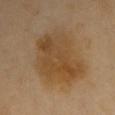notes = total-body-photography surveillance lesion; no biopsy
illumination = cross-polarized illumination
size = ≈8 mm
imaging modality = total-body-photography crop, ~15 mm field of view
body site = the front of the torso
patient = female, aged approximately 50